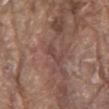{
  "biopsy_status": "not biopsied; imaged during a skin examination",
  "patient": {
    "sex": "male",
    "age_approx": 80
  },
  "site": "mid back",
  "image": {
    "source": "total-body photography crop",
    "field_of_view_mm": 15
  }
}